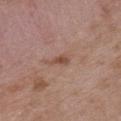Q: Is there a histopathology result?
A: no biopsy performed (imaged during a skin exam)
Q: How was the tile lit?
A: white-light
Q: How large is the lesion?
A: ≈2.5 mm
Q: What is the imaging modality?
A: ~15 mm crop, total-body skin-cancer survey
Q: Who is the patient?
A: male, aged around 50
Q: Where on the body is the lesion?
A: the chest